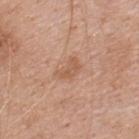Imaged during a routine full-body skin examination; the lesion was not biopsied and no histopathology is available. On the upper back. A 15 mm close-up extracted from a 3D total-body photography capture. A female subject, aged approximately 40. The lesion's longest dimension is about 3 mm.